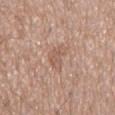Recorded during total-body skin imaging; not selected for excision or biopsy. The subject is a male approximately 75 years of age. A 15 mm close-up extracted from a 3D total-body photography capture. The total-body-photography lesion software estimated an eccentricity of roughly 0.85 and two-axis asymmetry of about 0.5. The software also gave an average lesion color of about L≈57 a*≈19 b*≈28 (CIELAB), roughly 7 lightness units darker than nearby skin, and a normalized lesion–skin contrast near 5. The software also gave a border-irregularity index near 4.5/10, internal color variation of about 2 on a 0–10 scale, and a peripheral color-asymmetry measure near 0.5. Imaged with white-light lighting. On the mid back. The recorded lesion diameter is about 4 mm.